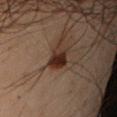Recorded during total-body skin imaging; not selected for excision or biopsy.
The lesion-visualizer software estimated a border-irregularity index near 2.5/10 and a peripheral color-asymmetry measure near 1.5.
Cropped from a total-body skin-imaging series; the visible field is about 15 mm.
The lesion's longest dimension is about 3.5 mm.
The lesion is located on the left upper arm.
A male patient aged approximately 50.